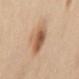The recorded lesion diameter is about 4 mm.
The patient is a male aged approximately 65.
Located on the back.
A 15 mm close-up extracted from a 3D total-body photography capture.